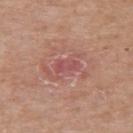| key | value |
|---|---|
| follow-up | no biopsy performed (imaged during a skin exam) |
| acquisition | ~15 mm crop, total-body skin-cancer survey |
| location | the left upper arm |
| automated lesion analysis | an area of roughly 3.5 mm², an outline eccentricity of about 0.9 (0 = round, 1 = elongated), and two-axis asymmetry of about 0.25; a classifier nevus-likeness of about 0/100 |
| patient | male, in their mid- to late 80s or older |
| lighting | white-light illumination |
| lesion size | ≈2.5 mm |